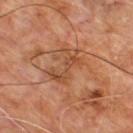biopsy status=imaged on a skin check; not biopsied
patient=male, aged 58–62
image=~15 mm tile from a whole-body skin photo
site=the upper back
tile lighting=cross-polarized illumination
automated metrics=an area of roughly 7 mm² and two-axis asymmetry of about 0.45; a lesion color around L≈46 a*≈23 b*≈34 in CIELAB, about 7 CIELAB-L* units darker than the surrounding skin, and a normalized border contrast of about 6; a border-irregularity index near 8/10, internal color variation of about 2 on a 0–10 scale, and peripheral color asymmetry of about 0.5; an automated nevus-likeness rating near 0 out of 100
diameter=~4.5 mm (longest diameter)